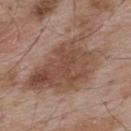No biopsy was performed on this lesion — it was imaged during a full skin examination and was not determined to be concerning.
Located on the upper back.
This image is a 15 mm lesion crop taken from a total-body photograph.
A male subject in their mid-50s.